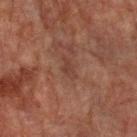Recorded during total-body skin imaging; not selected for excision or biopsy. Automated image analysis of the tile measured a lesion area of about 3 mm² and a symmetry-axis asymmetry near 0.4. The software also gave a lesion color around L≈32 a*≈18 b*≈22 in CIELAB, roughly 5 lightness units darker than nearby skin, and a lesion-to-skin contrast of about 5 (normalized; higher = more distinct). It also reported a border-irregularity index near 4/10 and a color-variation rating of about 1/10. Captured under cross-polarized illumination. The patient is a male aged around 75. Cropped from a total-body skin-imaging series; the visible field is about 15 mm. Located on the arm. Approximately 3 mm at its widest.